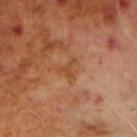| key | value |
|---|---|
| location | the right upper arm |
| tile lighting | cross-polarized illumination |
| image source | total-body-photography crop, ~15 mm field of view |
| subject | male, approximately 70 years of age |